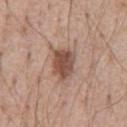  biopsy_status: not biopsied; imaged during a skin examination
  patient:
    sex: male
    age_approx: 55
  image:
    source: total-body photography crop
    field_of_view_mm: 15
  site: front of the torso
  lighting: white-light
  automated_metrics:
    eccentricity: 0.75
    shape_asymmetry: 0.2
    cielab_L: 50
    cielab_a: 20
    cielab_b: 26
    vs_skin_darker_L: 13.0
  lesion_size:
    long_diameter_mm_approx: 4.0